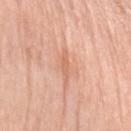Captured during whole-body skin photography for melanoma surveillance; the lesion was not biopsied.
Cropped from a whole-body photographic skin survey; the tile spans about 15 mm.
The patient is a female roughly 65 years of age.
On the left upper arm.